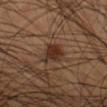site: leg
patient:
  sex: male
  age_approx: 45
image:
  source: total-body photography crop
  field_of_view_mm: 15